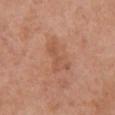Automated tile analysis of the lesion measured a lesion color around L≈55 a*≈23 b*≈32 in CIELAB and a lesion–skin lightness drop of about 6. The software also gave internal color variation of about 2.5 on a 0–10 scale and radial color variation of about 0.5. And it measured an automated nevus-likeness rating near 0 out of 100 and a lesion-detection confidence of about 100/100.
On the chest.
A female subject about 65 years old.
A 15 mm close-up extracted from a 3D total-body photography capture.
About 4.5 mm across.